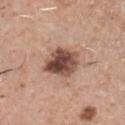This lesion was catalogued during total-body skin photography and was not selected for biopsy. About 4 mm across. Captured under white-light illumination. A 15 mm close-up tile from a total-body photography series done for melanoma screening. The lesion is located on the chest. A male subject in their mid- to late 40s.